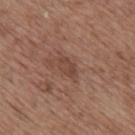Acquisition and patient details: The patient is a male roughly 70 years of age. Measured at roughly 3 mm in maximum diameter. The total-body-photography lesion software estimated an area of roughly 4 mm², an outline eccentricity of about 0.8 (0 = round, 1 = elongated), and two-axis asymmetry of about 0.25. And it measured a border-irregularity rating of about 2.5/10, internal color variation of about 2.5 on a 0–10 scale, and radial color variation of about 1. The analysis additionally found a detector confidence of about 100 out of 100 that the crop contains a lesion. A 15 mm close-up tile from a total-body photography series done for melanoma screening. Captured under white-light illumination. The lesion is located on the upper back.